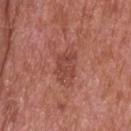Clinical impression: The lesion was photographed on a routine skin check and not biopsied; there is no pathology result. Context: On the head or neck. A male patient, roughly 65 years of age. Imaged with white-light lighting. Cropped from a total-body skin-imaging series; the visible field is about 15 mm. Measured at roughly 4 mm in maximum diameter.